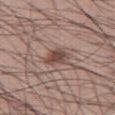workup=no biopsy performed (imaged during a skin exam) | lesion size=≈3 mm | site=the right thigh | subject=male, in their mid-50s | image source=~15 mm tile from a whole-body skin photo | illumination=white-light | image-analysis metrics=internal color variation of about 3.5 on a 0–10 scale and radial color variation of about 1; an automated nevus-likeness rating near 95 out of 100.